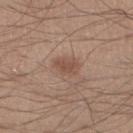<tbp_lesion>
<site>right lower leg</site>
<patient>
  <sex>male</sex>
  <age_approx>30</age_approx>
</patient>
<lesion_size>
  <long_diameter_mm_approx>3.0</long_diameter_mm_approx>
</lesion_size>
<image>
  <source>total-body photography crop</source>
  <field_of_view_mm>15</field_of_view_mm>
</image>
</tbp_lesion>